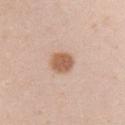notes=total-body-photography surveillance lesion; no biopsy
tile lighting=white-light illumination
site=the right upper arm
lesion size=~3 mm (longest diameter)
patient=female, in their 20s
image=total-body-photography crop, ~15 mm field of view